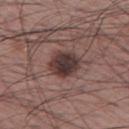Q: Is there a histopathology result?
A: total-body-photography surveillance lesion; no biopsy
Q: Where on the body is the lesion?
A: the left thigh
Q: What lighting was used for the tile?
A: white-light
Q: Patient demographics?
A: male, aged 58–62
Q: Lesion size?
A: about 4 mm
Q: What is the imaging modality?
A: ~15 mm crop, total-body skin-cancer survey